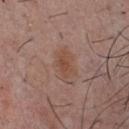Q: Was a biopsy performed?
A: imaged on a skin check; not biopsied
Q: Automated lesion metrics?
A: a lesion area of about 4.5 mm², a shape eccentricity near 0.85, and two-axis asymmetry of about 0.25; a nevus-likeness score of about 35/100 and a detector confidence of about 100 out of 100 that the crop contains a lesion
Q: What lighting was used for the tile?
A: white-light illumination
Q: Who is the patient?
A: male, roughly 55 years of age
Q: What is the lesion's diameter?
A: ≈3.5 mm
Q: Where on the body is the lesion?
A: the front of the torso
Q: How was this image acquired?
A: ~15 mm tile from a whole-body skin photo